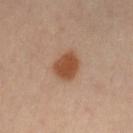Impression:
Imaged during a routine full-body skin examination; the lesion was not biopsied and no histopathology is available.
Image and clinical context:
A female patient aged 38 to 42. A 15 mm crop from a total-body photograph taken for skin-cancer surveillance. From the right leg.